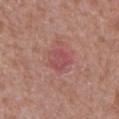lesion size = about 3 mm
tile lighting = white-light
location = the abdomen
patient = male, aged 63–67
image = total-body-photography crop, ~15 mm field of view
automated metrics = a footprint of about 7 mm², an eccentricity of roughly 0.5, and a symmetry-axis asymmetry near 0.2; a classifier nevus-likeness of about 0/100